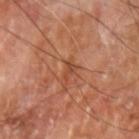Case summary:
– notes — total-body-photography surveillance lesion; no biopsy
– imaging modality — 15 mm crop, total-body photography
– illumination — cross-polarized illumination
– size — ≈3 mm
– patient — male, in their 70s
– anatomic site — the left upper arm